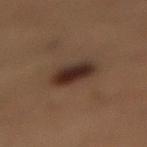notes: imaged on a skin check; not biopsied
diameter: ~4 mm (longest diameter)
body site: the lower back
imaging modality: ~15 mm crop, total-body skin-cancer survey
patient: male, approximately 50 years of age
tile lighting: cross-polarized illumination